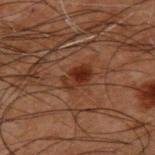<case>
  <biopsy_status>not biopsied; imaged during a skin examination</biopsy_status>
  <lighting>cross-polarized</lighting>
  <lesion_size>
    <long_diameter_mm_approx>3.5</long_diameter_mm_approx>
  </lesion_size>
  <site>back</site>
  <patient>
    <sex>male</sex>
    <age_approx>50</age_approx>
  </patient>
  <automated_metrics>
    <area_mm2_approx>6.0</area_mm2_approx>
    <shape_asymmetry>0.15</shape_asymmetry>
    <cielab_L>23</cielab_L>
    <cielab_a>19</cielab_a>
    <cielab_b>24</cielab_b>
    <vs_skin_darker_L>7.0</vs_skin_darker_L>
    <border_irregularity_0_10>2.0</border_irregularity_0_10>
    <color_variation_0_10>6.5</color_variation_0_10>
    <peripheral_color_asymmetry>2.5</peripheral_color_asymmetry>
    <lesion_detection_confidence_0_100>100</lesion_detection_confidence_0_100>
  </automated_metrics>
  <image>
    <source>total-body photography crop</source>
    <field_of_view_mm>15</field_of_view_mm>
  </image>
</case>